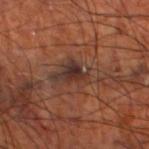Case summary:
- follow-up — catalogued during a skin exam; not biopsied
- lesion size — about 5.5 mm
- illumination — cross-polarized
- patient — male, aged 68–72
- image source — total-body-photography crop, ~15 mm field of view
- anatomic site — the left lower leg